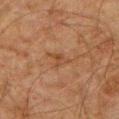notes = total-body-photography surveillance lesion; no biopsy | diameter = ≈2.5 mm | anatomic site = the chest | lighting = cross-polarized | patient = male, aged 58 to 62 | acquisition = total-body-photography crop, ~15 mm field of view.